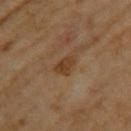workup — catalogued during a skin exam; not biopsied
subject — female, in their mid-60s
lighting — cross-polarized
acquisition — total-body-photography crop, ~15 mm field of view
automated metrics — a shape-asymmetry score of about 0.35 (0 = symmetric); a nevus-likeness score of about 10/100 and lesion-presence confidence of about 100/100
body site — the left upper arm